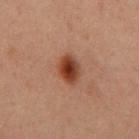Imaged during a routine full-body skin examination; the lesion was not biopsied and no histopathology is available. The total-body-photography lesion software estimated an area of roughly 7 mm² and a symmetry-axis asymmetry near 0.25. The software also gave a nevus-likeness score of about 100/100 and lesion-presence confidence of about 100/100. A lesion tile, about 15 mm wide, cut from a 3D total-body photograph. A male patient, in their 60s. The lesion is located on the chest.